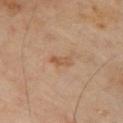Assessment: No biopsy was performed on this lesion — it was imaged during a full skin examination and was not determined to be concerning. Clinical summary: A region of skin cropped from a whole-body photographic capture, roughly 15 mm wide. Automated image analysis of the tile measured an area of roughly 3 mm² and a shape eccentricity near 0.9. And it measured an average lesion color of about L≈55 a*≈20 b*≈34 (CIELAB) and roughly 7 lightness units darker than nearby skin. The analysis additionally found an automated nevus-likeness rating near 0 out of 100 and lesion-presence confidence of about 100/100. The subject is a male aged around 65. From the chest. The recorded lesion diameter is about 2.5 mm. The tile uses cross-polarized illumination.